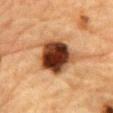biopsy status = catalogued during a skin exam; not biopsied | acquisition = ~15 mm crop, total-body skin-cancer survey | TBP lesion metrics = an automated nevus-likeness rating near 90 out of 100 and a lesion-detection confidence of about 100/100 | lesion diameter = about 5.5 mm | site = the chest | patient = male, roughly 85 years of age or older.